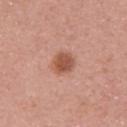A female patient, in their 40s. The lesion is on the right upper arm. This is a white-light tile. A 15 mm close-up tile from a total-body photography series done for melanoma screening. The lesion's longest dimension is about 3 mm.